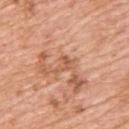Clinical impression:
Recorded during total-body skin imaging; not selected for excision or biopsy.
Image and clinical context:
On the upper back. Cropped from a total-body skin-imaging series; the visible field is about 15 mm. The patient is a female approximately 45 years of age. Automated tile analysis of the lesion measured a lesion area of about 3 mm², an outline eccentricity of about 0.8 (0 = round, 1 = elongated), and a symmetry-axis asymmetry near 0.6. It also reported a mean CIELAB color near L≈58 a*≈25 b*≈35, roughly 8 lightness units darker than nearby skin, and a lesion-to-skin contrast of about 6 (normalized; higher = more distinct). About 3 mm across.